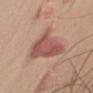Q: Was a biopsy performed?
A: no biopsy performed (imaged during a skin exam)
Q: Automated lesion metrics?
A: a lesion color around L≈54 a*≈25 b*≈26 in CIELAB and a normalized border contrast of about 8
Q: How was this image acquired?
A: ~15 mm tile from a whole-body skin photo
Q: What are the patient's age and sex?
A: male, aged approximately 50
Q: What is the anatomic site?
A: the left upper arm
Q: What lighting was used for the tile?
A: white-light illumination